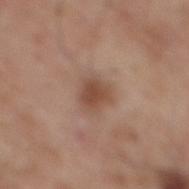workup: catalogued during a skin exam; not biopsied
location: the mid back
image source: total-body-photography crop, ~15 mm field of view
subject: male, approximately 60 years of age